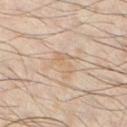follow-up — imaged on a skin check; not biopsied
subject — male, aged 33 to 37
automated metrics — an outline eccentricity of about 0.85 (0 = round, 1 = elongated); an automated nevus-likeness rating near 0 out of 100 and a lesion-detection confidence of about 95/100
illumination — cross-polarized
site — the chest
imaging modality — ~15 mm crop, total-body skin-cancer survey